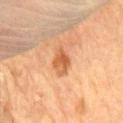follow-up — catalogued during a skin exam; not biopsied | illumination — cross-polarized | patient — male, aged 83 or older | size — ≈3.5 mm | anatomic site — the mid back | imaging modality — total-body-photography crop, ~15 mm field of view.